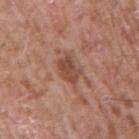workup = no biopsy performed (imaged during a skin exam)
lesion size = ≈3.5 mm
location = the right upper arm
image = ~15 mm crop, total-body skin-cancer survey
patient = male, aged approximately 55